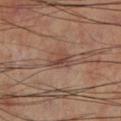workup: catalogued during a skin exam; not biopsied
site: the left lower leg
automated metrics: an eccentricity of roughly 0.7 and a symmetry-axis asymmetry near 0.3; a border-irregularity index near 3/10 and radial color variation of about 1.5; a lesion-detection confidence of about 100/100
image source: ~15 mm tile from a whole-body skin photo
size: ≈3 mm
illumination: cross-polarized illumination
subject: male, roughly 70 years of age